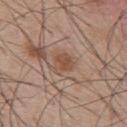Q: Is there a histopathology result?
A: imaged on a skin check; not biopsied
Q: How was this image acquired?
A: ~15 mm tile from a whole-body skin photo
Q: Who is the patient?
A: male, approximately 55 years of age
Q: What is the anatomic site?
A: the back
Q: What lighting was used for the tile?
A: white-light
Q: What is the lesion's diameter?
A: about 2.5 mm
Q: What did automated image analysis measure?
A: a lesion area of about 5 mm², a shape eccentricity near 0.5, and two-axis asymmetry of about 0.35; an automated nevus-likeness rating near 5 out of 100 and a lesion-detection confidence of about 100/100